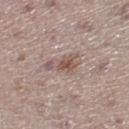The lesion is on the right lower leg. The lesion's longest dimension is about 4 mm. A female patient about 50 years old. Automated image analysis of the tile measured a footprint of about 7.5 mm² and a shape eccentricity near 0.85. The analysis additionally found a mean CIELAB color near L≈54 a*≈16 b*≈22 and about 9 CIELAB-L* units darker than the surrounding skin. The analysis additionally found an automated nevus-likeness rating near 45 out of 100 and a lesion-detection confidence of about 100/100. Captured under white-light illumination. A lesion tile, about 15 mm wide, cut from a 3D total-body photograph.